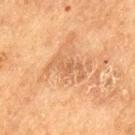This lesion was catalogued during total-body skin photography and was not selected for biopsy.
About 4.5 mm across.
Captured under cross-polarized illumination.
A male patient approximately 75 years of age.
A region of skin cropped from a whole-body photographic capture, roughly 15 mm wide.
The lesion is located on the left thigh.
The lesion-visualizer software estimated a footprint of about 7.5 mm², an eccentricity of roughly 0.9, and a shape-asymmetry score of about 0.5 (0 = symmetric). The software also gave internal color variation of about 2 on a 0–10 scale and peripheral color asymmetry of about 0.5.